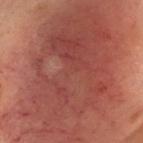Clinical impression: Imaged during a routine full-body skin examination; the lesion was not biopsied and no histopathology is available.